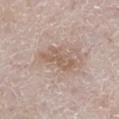Clinical impression:
The lesion was photographed on a routine skin check and not biopsied; there is no pathology result.
Background:
A male patient, aged 78 to 82. A 15 mm close-up extracted from a 3D total-body photography capture. Located on the left lower leg. An algorithmic analysis of the crop reported an area of roughly 7.5 mm² and a symmetry-axis asymmetry near 0.35. And it measured an average lesion color of about L≈58 a*≈16 b*≈26 (CIELAB), a lesion–skin lightness drop of about 9, and a normalized border contrast of about 6.5. It also reported border irregularity of about 4.5 on a 0–10 scale and internal color variation of about 2 on a 0–10 scale. And it measured an automated nevus-likeness rating near 0 out of 100 and lesion-presence confidence of about 100/100.